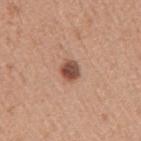This lesion was catalogued during total-body skin photography and was not selected for biopsy.
Automated tile analysis of the lesion measured a lesion–skin lightness drop of about 15 and a normalized lesion–skin contrast near 10.5. The software also gave a nevus-likeness score of about 95/100 and lesion-presence confidence of about 100/100.
A male subject, aged approximately 40.
On the arm.
A 15 mm crop from a total-body photograph taken for skin-cancer surveillance.
Approximately 2.5 mm at its widest.
This is a white-light tile.